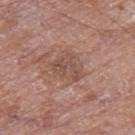Q: Was this lesion biopsied?
A: catalogued during a skin exam; not biopsied
Q: How was the tile lit?
A: white-light
Q: Who is the patient?
A: male, approximately 80 years of age
Q: What is the imaging modality?
A: total-body-photography crop, ~15 mm field of view
Q: What is the lesion's diameter?
A: ≈3.5 mm
Q: Where on the body is the lesion?
A: the right thigh